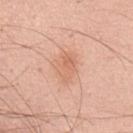Impression:
The lesion was photographed on a routine skin check and not biopsied; there is no pathology result.
Acquisition and patient details:
The subject is a male aged 48 to 52. This is a white-light tile. A 15 mm close-up extracted from a 3D total-body photography capture. From the front of the torso. Automated tile analysis of the lesion measured an area of roughly 6.5 mm², a shape eccentricity near 0.75, and a symmetry-axis asymmetry near 0.2. The analysis additionally found a lesion color around L≈66 a*≈24 b*≈33 in CIELAB and a normalized lesion–skin contrast near 5. It also reported a peripheral color-asymmetry measure near 1.5. The recorded lesion diameter is about 3.5 mm.